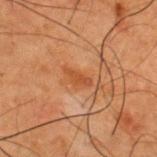Assessment: The lesion was photographed on a routine skin check and not biopsied; there is no pathology result.